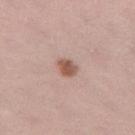Clinical impression: No biopsy was performed on this lesion — it was imaged during a full skin examination and was not determined to be concerning. Image and clinical context: A female patient aged 38–42. A roughly 15 mm field-of-view crop from a total-body skin photograph. Located on the left thigh.